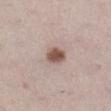workup: imaged on a skin check; not biopsied | anatomic site: the right lower leg | automated metrics: an area of roughly 5 mm², a shape eccentricity near 0.7, and a shape-asymmetry score of about 0.15 (0 = symmetric); a mean CIELAB color near L≈53 a*≈18 b*≈24 and a lesion–skin lightness drop of about 15; a border-irregularity index near 1/10, a color-variation rating of about 2.5/10, and a peripheral color-asymmetry measure near 1; a classifier nevus-likeness of about 95/100 and a lesion-detection confidence of about 100/100 | tile lighting: white-light | patient: female, aged around 40 | acquisition: ~15 mm tile from a whole-body skin photo.